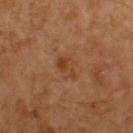Impression:
The lesion was photographed on a routine skin check and not biopsied; there is no pathology result.
Background:
Longest diameter approximately 3 mm. A male subject, approximately 60 years of age. A lesion tile, about 15 mm wide, cut from a 3D total-body photograph. From the upper back. Imaged with cross-polarized lighting.